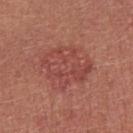biopsy status = total-body-photography surveillance lesion; no biopsy | lighting = white-light | subject = female, aged 23 to 27 | anatomic site = the left upper arm | image = total-body-photography crop, ~15 mm field of view.